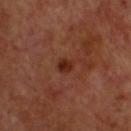The lesion was photographed on a routine skin check and not biopsied; there is no pathology result.